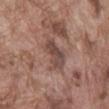This lesion was catalogued during total-body skin photography and was not selected for biopsy. A male patient, aged approximately 75. The lesion is on the abdomen. A 15 mm close-up tile from a total-body photography series done for melanoma screening. Captured under white-light illumination. The total-body-photography lesion software estimated a lesion–skin lightness drop of about 10 and a normalized lesion–skin contrast near 7.5. And it measured border irregularity of about 4.5 on a 0–10 scale, a color-variation rating of about 4.5/10, and radial color variation of about 1.5.